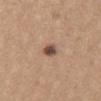biopsy status = total-body-photography surveillance lesion; no biopsy | patient = female, about 35 years old | image = total-body-photography crop, ~15 mm field of view | anatomic site = the mid back.